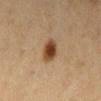Clinical impression: Recorded during total-body skin imaging; not selected for excision or biopsy. Acquisition and patient details: The tile uses cross-polarized illumination. Longest diameter approximately 3 mm. This image is a 15 mm lesion crop taken from a total-body photograph. The patient is a female approximately 40 years of age. The lesion is on the leg. An algorithmic analysis of the crop reported border irregularity of about 2 on a 0–10 scale and a peripheral color-asymmetry measure near 1. The software also gave an automated nevus-likeness rating near 100 out of 100 and lesion-presence confidence of about 100/100.